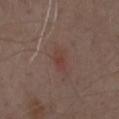A male subject aged 68–72.
A lesion tile, about 15 mm wide, cut from a 3D total-body photograph.
The lesion is located on the chest.